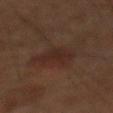notes: catalogued during a skin exam; not biopsied | illumination: cross-polarized illumination | size: ~3.5 mm (longest diameter) | automated metrics: a symmetry-axis asymmetry near 0.4; a border-irregularity rating of about 4/10, a within-lesion color-variation index near 1.5/10, and a peripheral color-asymmetry measure near 0.5 | patient: male, roughly 60 years of age | site: the mid back | imaging modality: ~15 mm crop, total-body skin-cancer survey.